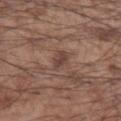| field | value |
|---|---|
| notes | catalogued during a skin exam; not biopsied |
| lighting | white-light |
| image source | 15 mm crop, total-body photography |
| patient | male, roughly 55 years of age |
| size | about 3 mm |
| location | the left forearm |
| automated metrics | a lesion area of about 3.5 mm², a shape eccentricity near 0.85, and two-axis asymmetry of about 0.3; internal color variation of about 1.5 on a 0–10 scale and peripheral color asymmetry of about 0.5; an automated nevus-likeness rating near 0 out of 100 and a lesion-detection confidence of about 95/100 |